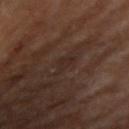Clinical impression:
Part of a total-body skin-imaging series; this lesion was reviewed on a skin check and was not flagged for biopsy.
Context:
A male subject in their mid-80s or older. Measured at roughly 3 mm in maximum diameter. On the arm. A region of skin cropped from a whole-body photographic capture, roughly 15 mm wide. An algorithmic analysis of the crop reported a footprint of about 3.5 mm², an outline eccentricity of about 0.85 (0 = round, 1 = elongated), and a symmetry-axis asymmetry near 0.45. The software also gave border irregularity of about 4.5 on a 0–10 scale, a within-lesion color-variation index near 1/10, and radial color variation of about 0.5.